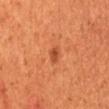Part of a total-body skin-imaging series; this lesion was reviewed on a skin check and was not flagged for biopsy.
The lesion's longest dimension is about 2 mm.
A lesion tile, about 15 mm wide, cut from a 3D total-body photograph.
The lesion is located on the mid back.
The subject is a male roughly 45 years of age.